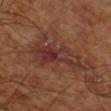Recorded during total-body skin imaging; not selected for excision or biopsy. The recorded lesion diameter is about 6.5 mm. Captured under cross-polarized illumination. A 15 mm crop from a total-body photograph taken for skin-cancer surveillance. From the right lower leg. A subject in their mid- to late 60s. Automated tile analysis of the lesion measured a lesion color around L≈32 a*≈22 b*≈24 in CIELAB, about 7 CIELAB-L* units darker than the surrounding skin, and a normalized lesion–skin contrast near 7.5.